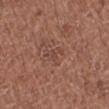Case summary:
- notes — catalogued during a skin exam; not biopsied
- subject — female, aged around 50
- location — the right lower leg
- acquisition — ~15 mm crop, total-body skin-cancer survey